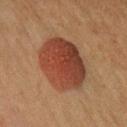biopsy status: catalogued during a skin exam; not biopsied | location: the left upper arm | image: ~15 mm tile from a whole-body skin photo | tile lighting: cross-polarized | lesion diameter: about 6.5 mm | image-analysis metrics: an area of roughly 27 mm² and an outline eccentricity of about 0.45 (0 = round, 1 = elongated); roughly 11 lightness units darker than nearby skin and a normalized lesion–skin contrast near 10; a classifier nevus-likeness of about 100/100 and lesion-presence confidence of about 100/100 | patient: female, in their 50s.